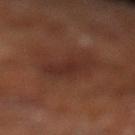Clinical impression:
Captured during whole-body skin photography for melanoma surveillance; the lesion was not biopsied.
Image and clinical context:
A 15 mm crop from a total-body photograph taken for skin-cancer surveillance. A male subject, in their 70s. This is a cross-polarized tile. Located on the left lower leg.